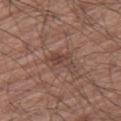Notes:
- image-analysis metrics: an area of roughly 4.5 mm², a shape eccentricity near 0.8, and a symmetry-axis asymmetry near 0.4
- subject: male, aged around 75
- anatomic site: the right thigh
- tile lighting: white-light
- acquisition: ~15 mm crop, total-body skin-cancer survey